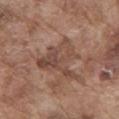workup = no biopsy performed (imaged during a skin exam) | size = ~6.5 mm (longest diameter) | body site = the mid back | patient = male, aged approximately 75 | acquisition = ~15 mm tile from a whole-body skin photo.